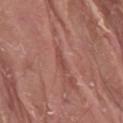The lesion was photographed on a routine skin check and not biopsied; there is no pathology result.
Measured at roughly 2.5 mm in maximum diameter.
Imaged with white-light lighting.
A 15 mm crop from a total-body photograph taken for skin-cancer surveillance.
From the lower back.
The subject is a male aged around 40.